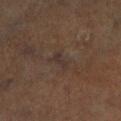Recorded during total-body skin imaging; not selected for excision or biopsy. A male subject, in their 60s. A roughly 15 mm field-of-view crop from a total-body skin photograph. From the leg. This is a cross-polarized tile.